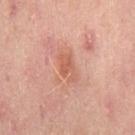| key | value |
|---|---|
| biopsy status | imaged on a skin check; not biopsied |
| imaging modality | ~15 mm tile from a whole-body skin photo |
| patient | female, in their 50s |
| location | the left thigh |